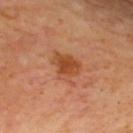biopsy status — total-body-photography surveillance lesion; no biopsy.